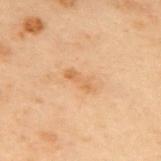Q: Was a biopsy performed?
A: no biopsy performed (imaged during a skin exam)
Q: Lesion size?
A: ≈3.5 mm
Q: What kind of image is this?
A: ~15 mm tile from a whole-body skin photo
Q: Who is the patient?
A: male, in their mid- to late 50s
Q: Illumination type?
A: cross-polarized
Q: What is the anatomic site?
A: the upper back